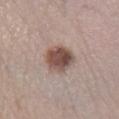  biopsy_status: not biopsied; imaged during a skin examination
  lesion_size:
    long_diameter_mm_approx: 4.0
  patient:
    sex: male
    age_approx: 45
  lighting: white-light
  image:
    source: total-body photography crop
    field_of_view_mm: 15
  automated_metrics:
    area_mm2_approx: 10.0
    eccentricity: 0.5
    shape_asymmetry: 0.2
    cielab_L: 49
    cielab_a: 17
    cielab_b: 22
    vs_skin_darker_L: 15.0
    vs_skin_contrast_norm: 10.5
    color_variation_0_10: 5.5
    peripheral_color_asymmetry: 2.0
    nevus_likeness_0_100: 70
  site: left lower leg